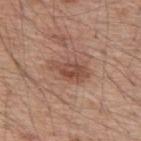Findings:
– workup · no biopsy performed (imaged during a skin exam)
– image · ~15 mm tile from a whole-body skin photo
– patient · male, aged 53–57
– location · the upper back
– illumination · white-light